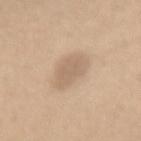biopsy status=imaged on a skin check; not biopsied
tile lighting=white-light
location=the mid back
acquisition=~15 mm crop, total-body skin-cancer survey
automated lesion analysis=an average lesion color of about L≈63 a*≈15 b*≈30 (CIELAB) and a normalized lesion–skin contrast near 5.5; an automated nevus-likeness rating near 30 out of 100 and lesion-presence confidence of about 100/100
subject=female, aged 33–37